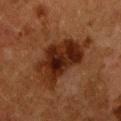{"biopsy_status": "not biopsied; imaged during a skin examination", "automated_metrics": {"area_mm2_approx": 25.0, "eccentricity": 0.85, "shape_asymmetry": 0.35, "cielab_L": 22, "cielab_a": 20, "cielab_b": 25, "vs_skin_darker_L": 10.0, "vs_skin_contrast_norm": 11.0, "color_variation_0_10": 7.0, "peripheral_color_asymmetry": 2.5}, "patient": {"sex": "female", "age_approx": 50}, "lesion_size": {"long_diameter_mm_approx": 9.0}, "image": {"source": "total-body photography crop", "field_of_view_mm": 15}, "site": "front of the torso"}